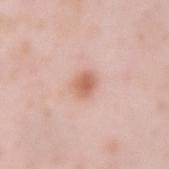Assessment:
Part of a total-body skin-imaging series; this lesion was reviewed on a skin check and was not flagged for biopsy.
Clinical summary:
The subject is a female aged around 40. Cropped from a whole-body photographic skin survey; the tile spans about 15 mm. An algorithmic analysis of the crop reported a border-irregularity rating of about 1.5/10, a color-variation rating of about 3/10, and radial color variation of about 1. From the left forearm. Imaged with white-light lighting.